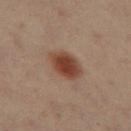notes: no biopsy performed (imaged during a skin exam) | tile lighting: cross-polarized illumination | subject: female, aged around 40 | acquisition: 15 mm crop, total-body photography | lesion size: ~4.5 mm (longest diameter) | automated metrics: a lesion area of about 9.5 mm² and a shape-asymmetry score of about 0.15 (0 = symmetric); an average lesion color of about L≈44 a*≈21 b*≈28 (CIELAB); a lesion-detection confidence of about 100/100 | body site: the leg.